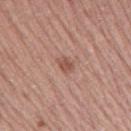* notes — total-body-photography surveillance lesion; no biopsy
* patient — male, aged 53 to 57
* image source — 15 mm crop, total-body photography
* image-analysis metrics — a mean CIELAB color near L≈53 a*≈22 b*≈27 and a normalized lesion–skin contrast near 6.5; a classifier nevus-likeness of about 45/100 and a lesion-detection confidence of about 100/100
* site — the right upper arm